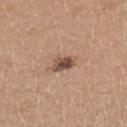Q: Was this lesion biopsied?
A: no biopsy performed (imaged during a skin exam)
Q: What kind of image is this?
A: 15 mm crop, total-body photography
Q: What are the patient's age and sex?
A: female, aged approximately 35
Q: Lesion location?
A: the left lower leg